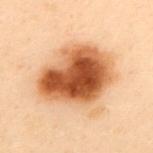follow-up: catalogued during a skin exam; not biopsied
illumination: cross-polarized
lesion diameter: ~7.5 mm (longest diameter)
subject: female, aged 58 to 62
location: the upper back
imaging modality: 15 mm crop, total-body photography